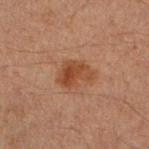| feature | finding |
|---|---|
| follow-up | total-body-photography surveillance lesion; no biopsy |
| diameter | about 4 mm |
| anatomic site | the leg |
| subject | male, approximately 30 years of age |
| image | ~15 mm crop, total-body skin-cancer survey |